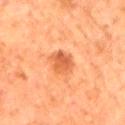Case summary:
– follow-up: no biopsy performed (imaged during a skin exam)
– image: 15 mm crop, total-body photography
– location: the lower back
– subject: male, aged around 80
– image-analysis metrics: a lesion area of about 6 mm², an outline eccentricity of about 0.45 (0 = round, 1 = elongated), and a symmetry-axis asymmetry near 0.25; a classifier nevus-likeness of about 65/100